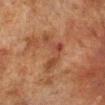follow-up — catalogued during a skin exam; not biopsied | acquisition — ~15 mm tile from a whole-body skin photo | lesion diameter — ≈5.5 mm | patient — male, aged 68–72 | illumination — cross-polarized | body site — the right lower leg | image-analysis metrics — a mean CIELAB color near L≈38 a*≈21 b*≈28, about 6 CIELAB-L* units darker than the surrounding skin, and a lesion-to-skin contrast of about 5.5 (normalized; higher = more distinct).